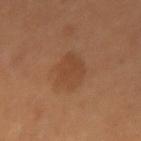Clinical impression:
Recorded during total-body skin imaging; not selected for excision or biopsy.
Acquisition and patient details:
The recorded lesion diameter is about 4.5 mm. A female subject aged 33–37. A roughly 15 mm field-of-view crop from a total-body skin photograph. On the left forearm.